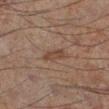Recorded during total-body skin imaging; not selected for excision or biopsy.
A 15 mm crop from a total-body photograph taken for skin-cancer surveillance.
A male patient approximately 60 years of age.
The lesion is on the left lower leg.